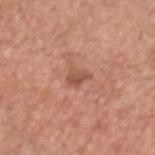Acquisition and patient details: Imaged with white-light lighting. A male subject, about 75 years old. The recorded lesion diameter is about 2.5 mm. On the head or neck. A 15 mm crop from a total-body photograph taken for skin-cancer surveillance.